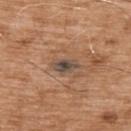No biopsy was performed on this lesion — it was imaged during a full skin examination and was not determined to be concerning. The patient is a male aged around 75. The lesion is on the upper back. The total-body-photography lesion software estimated a mean CIELAB color near L≈47 a*≈14 b*≈25, a lesion–skin lightness drop of about 10, and a lesion-to-skin contrast of about 9.5 (normalized; higher = more distinct). This is a white-light tile. Approximately 3.5 mm at its widest. A 15 mm close-up extracted from a 3D total-body photography capture.